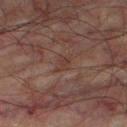Part of a total-body skin-imaging series; this lesion was reviewed on a skin check and was not flagged for biopsy.
A lesion tile, about 15 mm wide, cut from a 3D total-body photograph.
Longest diameter approximately 3 mm.
The patient is a male aged 73–77.
The lesion is located on the right thigh.
The tile uses cross-polarized illumination.
The lesion-visualizer software estimated a classifier nevus-likeness of about 0/100.